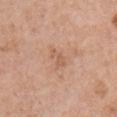Part of a total-body skin-imaging series; this lesion was reviewed on a skin check and was not flagged for biopsy.
Imaged with white-light lighting.
Automated image analysis of the tile measured an average lesion color of about L≈59 a*≈22 b*≈32 (CIELAB), roughly 7 lightness units darker than nearby skin, and a lesion-to-skin contrast of about 4.5 (normalized; higher = more distinct). And it measured a border-irregularity index near 4.5/10 and internal color variation of about 0.5 on a 0–10 scale. The software also gave an automated nevus-likeness rating near 0 out of 100.
A female patient approximately 50 years of age.
The lesion is on the chest.
A region of skin cropped from a whole-body photographic capture, roughly 15 mm wide.
Longest diameter approximately 2.5 mm.